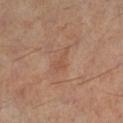<case>
<biopsy_status>not biopsied; imaged during a skin examination</biopsy_status>
<lesion_size>
  <long_diameter_mm_approx>3.0</long_diameter_mm_approx>
</lesion_size>
<automated_metrics>
  <area_mm2_approx>3.0</area_mm2_approx>
  <eccentricity>0.9</eccentricity>
  <shape_asymmetry>0.6</shape_asymmetry>
  <border_irregularity_0_10>6.5</border_irregularity_0_10>
  <color_variation_0_10>0.0</color_variation_0_10>
  <peripheral_color_asymmetry>0.0</peripheral_color_asymmetry>
</automated_metrics>
<image>
  <source>total-body photography crop</source>
  <field_of_view_mm>15</field_of_view_mm>
</image>
<patient>
  <sex>male</sex>
  <age_approx>60</age_approx>
</patient>
<lighting>cross-polarized</lighting>
<site>left lower leg</site>
</case>